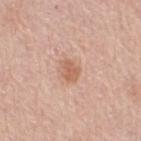follow-up: total-body-photography surveillance lesion; no biopsy | anatomic site: the right thigh | acquisition: ~15 mm crop, total-body skin-cancer survey | lighting: white-light illumination | automated metrics: an area of roughly 4 mm², an eccentricity of roughly 0.6, and a symmetry-axis asymmetry near 0.3; a lesion color around L≈61 a*≈23 b*≈31 in CIELAB, roughly 9 lightness units darker than nearby skin, and a normalized border contrast of about 6.5; a classifier nevus-likeness of about 55/100 and a detector confidence of about 100 out of 100 that the crop contains a lesion | patient: female, aged 63–67.